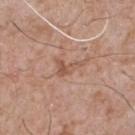notes: imaged on a skin check; not biopsied | anatomic site: the chest | patient: male, aged around 75 | size: ~3.5 mm (longest diameter) | lighting: white-light illumination | image-analysis metrics: a footprint of about 4 mm², an outline eccentricity of about 0.85 (0 = round, 1 = elongated), and a symmetry-axis asymmetry near 0.65; a peripheral color-asymmetry measure near 0.5; a nevus-likeness score of about 0/100 and lesion-presence confidence of about 100/100 | image source: ~15 mm crop, total-body skin-cancer survey.